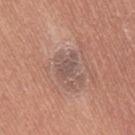{"biopsy_status": "not biopsied; imaged during a skin examination", "patient": {"sex": "female", "age_approx": 55}, "automated_metrics": {"area_mm2_approx": 4.0, "eccentricity": 0.8, "shape_asymmetry": 0.45, "peripheral_color_asymmetry": 0.5, "nevus_likeness_0_100": 0}, "lesion_size": {"long_diameter_mm_approx": 3.0}, "image": {"source": "total-body photography crop", "field_of_view_mm": 15}, "site": "right thigh", "lighting": "white-light"}